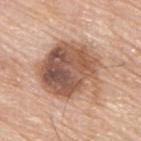Part of a total-body skin-imaging series; this lesion was reviewed on a skin check and was not flagged for biopsy.
The lesion is on the upper back.
A male subject, about 80 years old.
A roughly 15 mm field-of-view crop from a total-body skin photograph.